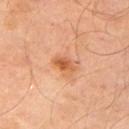The lesion was photographed on a routine skin check and not biopsied; there is no pathology result.
Cropped from a total-body skin-imaging series; the visible field is about 15 mm.
Located on the left upper arm.
A male subject, in their mid-60s.